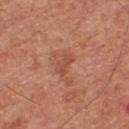Clinical impression: Recorded during total-body skin imaging; not selected for excision or biopsy. Background: A male subject, aged 63–67. The recorded lesion diameter is about 2.5 mm. Located on the front of the torso. A roughly 15 mm field-of-view crop from a total-body skin photograph.